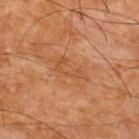| feature | finding |
|---|---|
| notes | no biopsy performed (imaged during a skin exam) |
| imaging modality | 15 mm crop, total-body photography |
| automated lesion analysis | a lesion–skin lightness drop of about 5; border irregularity of about 3 on a 0–10 scale, internal color variation of about 3 on a 0–10 scale, and a peripheral color-asymmetry measure near 1 |
| patient | male, in their mid- to late 60s |
| anatomic site | the upper back |